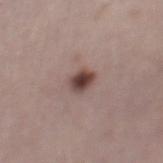From the left lower leg. A roughly 15 mm field-of-view crop from a total-body skin photograph. The patient is a female approximately 50 years of age. The tile uses white-light illumination.